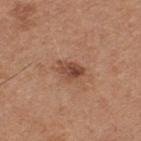Assessment: Imaged during a routine full-body skin examination; the lesion was not biopsied and no histopathology is available. Acquisition and patient details: The lesion-visualizer software estimated a lesion area of about 5.5 mm², a shape eccentricity near 0.75, and two-axis asymmetry of about 0.3. It also reported a lesion color around L≈47 a*≈22 b*≈30 in CIELAB, about 11 CIELAB-L* units darker than the surrounding skin, and a normalized lesion–skin contrast near 8. The analysis additionally found a color-variation rating of about 6/10 and a peripheral color-asymmetry measure near 2.5. Imaged with white-light lighting. On the upper back. The lesion's longest dimension is about 3.5 mm. A male patient roughly 65 years of age. A 15 mm crop from a total-body photograph taken for skin-cancer surveillance.